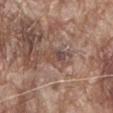{"biopsy_status": "not biopsied; imaged during a skin examination", "image": {"source": "total-body photography crop", "field_of_view_mm": 15}, "patient": {"sex": "male", "age_approx": 80}, "site": "abdomen"}